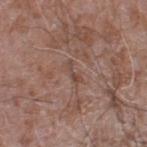biopsy status = total-body-photography surveillance lesion; no biopsy
size = about 2.5 mm
patient = male, aged 58–62
image = ~15 mm tile from a whole-body skin photo
body site = the left lower leg